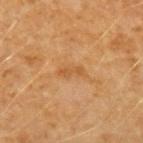Findings:
– follow-up — no biopsy performed (imaged during a skin exam)
– tile lighting — cross-polarized illumination
– patient — female, aged around 50
– anatomic site — the left forearm
– acquisition — ~15 mm crop, total-body skin-cancer survey
– lesion size — about 3 mm
– image-analysis metrics — an area of roughly 2.5 mm² and a shape eccentricity near 0.9; about 7 CIELAB-L* units darker than the surrounding skin